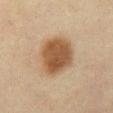This lesion was catalogued during total-body skin photography and was not selected for biopsy.
This is a cross-polarized tile.
A 15 mm crop from a total-body photograph taken for skin-cancer surveillance.
Located on the chest.
A female subject aged 58–62.
The lesion-visualizer software estimated a lesion area of about 19 mm². It also reported border irregularity of about 1.5 on a 0–10 scale, a within-lesion color-variation index near 3.5/10, and peripheral color asymmetry of about 1. And it measured a nevus-likeness score of about 100/100 and a detector confidence of about 100 out of 100 that the crop contains a lesion.